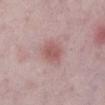Assessment:
This lesion was catalogued during total-body skin photography and was not selected for biopsy.
Context:
A lesion tile, about 15 mm wide, cut from a 3D total-body photograph. Approximately 3 mm at its widest. Located on the left lower leg. Captured under white-light illumination. A female subject aged approximately 45.